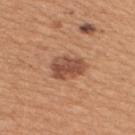Case summary:
- patient: female, aged 28 to 32
- image: ~15 mm crop, total-body skin-cancer survey
- illumination: white-light illumination
- body site: the upper back
- image-analysis metrics: a border-irregularity index near 2.5/10, internal color variation of about 4 on a 0–10 scale, and a peripheral color-asymmetry measure near 1.5; lesion-presence confidence of about 100/100
- size: ≈4.5 mm